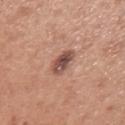Clinical impression: This lesion was catalogued during total-body skin photography and was not selected for biopsy. Clinical summary: A female subject, roughly 30 years of age. The tile uses white-light illumination. The lesion is on the left upper arm. A roughly 15 mm field-of-view crop from a total-body skin photograph.